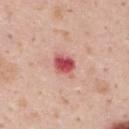{
  "lesion_size": {
    "long_diameter_mm_approx": 2.5
  },
  "patient": {
    "sex": "male",
    "age_approx": 35
  },
  "automated_metrics": {
    "eccentricity": 0.45,
    "shape_asymmetry": 0.15
  },
  "site": "upper back",
  "image": {
    "source": "total-body photography crop",
    "field_of_view_mm": 15
  },
  "lighting": "white-light"
}